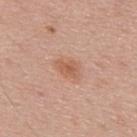workup — total-body-photography surveillance lesion; no biopsy
subject — male, aged 43–47
acquisition — ~15 mm tile from a whole-body skin photo
location — the upper back
lesion size — about 3 mm
TBP lesion metrics — a shape eccentricity near 0.8 and a symmetry-axis asymmetry near 0.25; a mean CIELAB color near L≈58 a*≈23 b*≈32, a lesion–skin lightness drop of about 8, and a normalized lesion–skin contrast near 6.5; a border-irregularity rating of about 2.5/10, a within-lesion color-variation index near 2/10, and peripheral color asymmetry of about 0.5; lesion-presence confidence of about 100/100